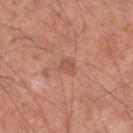| field | value |
|---|---|
| imaging modality | 15 mm crop, total-body photography |
| automated metrics | an area of roughly 4 mm², an outline eccentricity of about 0.7 (0 = round, 1 = elongated), and a symmetry-axis asymmetry near 0.3; a lesion color around L≈55 a*≈24 b*≈31 in CIELAB and a normalized lesion–skin contrast near 4.5; a border-irregularity rating of about 2.5/10, a within-lesion color-variation index near 2/10, and a peripheral color-asymmetry measure near 0.5; a nevus-likeness score of about 0/100 |
| size | about 3 mm |
| subject | male, in their mid-50s |
| illumination | white-light |
| location | the left lower leg |